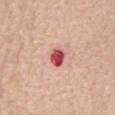Q: Was this lesion biopsied?
A: no biopsy performed (imaged during a skin exam)
Q: Lesion location?
A: the abdomen
Q: Who is the patient?
A: female, aged 68 to 72
Q: What is the imaging modality?
A: total-body-photography crop, ~15 mm field of view